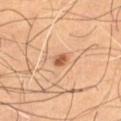- workup · no biopsy performed (imaged during a skin exam)
- subject · male, about 65 years old
- imaging modality · 15 mm crop, total-body photography
- location · the right thigh